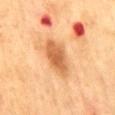No biopsy was performed on this lesion — it was imaged during a full skin examination and was not determined to be concerning. On the mid back. Automated image analysis of the tile measured a lesion–skin lightness drop of about 12. It also reported a color-variation rating of about 3.5/10 and peripheral color asymmetry of about 1. And it measured a classifier nevus-likeness of about 0/100 and a lesion-detection confidence of about 100/100. A female patient approximately 55 years of age. Longest diameter approximately 5 mm. This is a cross-polarized tile. A lesion tile, about 15 mm wide, cut from a 3D total-body photograph.